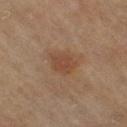Impression:
This lesion was catalogued during total-body skin photography and was not selected for biopsy.
Image and clinical context:
The lesion is on the right thigh. A 15 mm close-up tile from a total-body photography series done for melanoma screening. Measured at roughly 3.5 mm in maximum diameter. A female subject approximately 60 years of age.